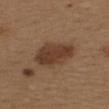Clinical impression:
Imaged during a routine full-body skin examination; the lesion was not biopsied and no histopathology is available.
Clinical summary:
The patient is a female about 40 years old. An algorithmic analysis of the crop reported a lesion color around L≈39 a*≈18 b*≈28 in CIELAB, about 11 CIELAB-L* units darker than the surrounding skin, and a normalized border contrast of about 9. Cropped from a whole-body photographic skin survey; the tile spans about 15 mm. About 5.5 mm across. On the upper back. This is a white-light tile.